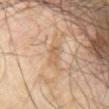notes: no biopsy performed (imaged during a skin exam) | imaging modality: ~15 mm tile from a whole-body skin photo | subject: male, aged 63–67 | location: the front of the torso | illumination: cross-polarized | size: about 2.5 mm.